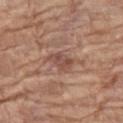Part of a total-body skin-imaging series; this lesion was reviewed on a skin check and was not flagged for biopsy. Longest diameter approximately 3 mm. A close-up tile cropped from a whole-body skin photograph, about 15 mm across. An algorithmic analysis of the crop reported an area of roughly 5 mm², a shape eccentricity near 0.8, and a shape-asymmetry score of about 0.3 (0 = symmetric). The software also gave a lesion color around L≈49 a*≈21 b*≈26 in CIELAB, about 9 CIELAB-L* units darker than the surrounding skin, and a normalized lesion–skin contrast near 6.5. It also reported a within-lesion color-variation index near 3/10 and peripheral color asymmetry of about 1. The software also gave a classifier nevus-likeness of about 0/100. A female subject roughly 75 years of age. Captured under white-light illumination. On the left thigh.